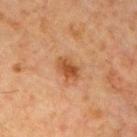Assessment: Recorded during total-body skin imaging; not selected for excision or biopsy. Acquisition and patient details: On the chest. The lesion's longest dimension is about 3 mm. A male patient, aged approximately 65. A lesion tile, about 15 mm wide, cut from a 3D total-body photograph. Automated image analysis of the tile measured a footprint of about 4.5 mm² and a symmetry-axis asymmetry near 0.2. The analysis additionally found a nevus-likeness score of about 85/100.